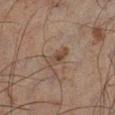Findings:
* workup: no biopsy performed (imaged during a skin exam)
* location: the left leg
* lighting: cross-polarized
* automated lesion analysis: a border-irregularity index near 3.5/10 and a color-variation rating of about 3.5/10; a classifier nevus-likeness of about 5/100 and lesion-presence confidence of about 100/100
* acquisition: ~15 mm tile from a whole-body skin photo
* patient: male, in their 60s